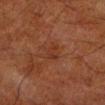| key | value |
|---|---|
| workup | catalogued during a skin exam; not biopsied |
| lesion size | ~2.5 mm (longest diameter) |
| body site | the left lower leg |
| tile lighting | cross-polarized illumination |
| subject | male, in their 80s |
| imaging modality | total-body-photography crop, ~15 mm field of view |